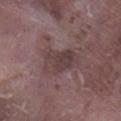• notes: total-body-photography surveillance lesion; no biopsy
• subject: male, aged 73–77
• location: the right lower leg
• acquisition: 15 mm crop, total-body photography